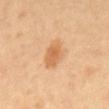follow-up: total-body-photography surveillance lesion; no biopsy
automated lesion analysis: border irregularity of about 2.5 on a 0–10 scale, internal color variation of about 3 on a 0–10 scale, and peripheral color asymmetry of about 1; a nevus-likeness score of about 90/100
acquisition: 15 mm crop, total-body photography
illumination: cross-polarized
diameter: ~4 mm (longest diameter)
subject: male, approximately 65 years of age
body site: the abdomen